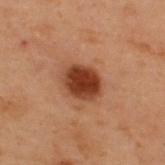{
  "biopsy_status": "not biopsied; imaged during a skin examination",
  "patient": {
    "sex": "male",
    "age_approx": 55
  },
  "lighting": "cross-polarized",
  "site": "back",
  "automated_metrics": {
    "area_mm2_approx": 9.0,
    "eccentricity": 0.6,
    "shape_asymmetry": 0.1,
    "cielab_L": 31,
    "cielab_a": 23,
    "cielab_b": 28,
    "vs_skin_darker_L": 14.0,
    "vs_skin_contrast_norm": 12.5,
    "nevus_likeness_0_100": 100,
    "lesion_detection_confidence_0_100": 100
  },
  "image": {
    "source": "total-body photography crop",
    "field_of_view_mm": 15
  }
}